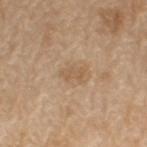notes = imaged on a skin check; not biopsied
anatomic site = the arm
imaging modality = ~15 mm tile from a whole-body skin photo
patient = male, roughly 70 years of age